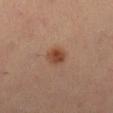biopsy status: catalogued during a skin exam; not biopsied
body site: the right lower leg
tile lighting: cross-polarized
subject: female, aged around 35
image: ~15 mm tile from a whole-body skin photo